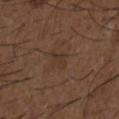A male subject, aged 48 to 52. On the chest. This is a white-light tile. Automated tile analysis of the lesion measured an outline eccentricity of about 0.7 (0 = round, 1 = elongated). Cropped from a whole-body photographic skin survey; the tile spans about 15 mm. The recorded lesion diameter is about 2.5 mm.